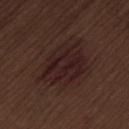The lesion was photographed on a routine skin check and not biopsied; there is no pathology result. The lesion-visualizer software estimated a footprint of about 20 mm², a shape eccentricity near 0.65, and two-axis asymmetry of about 0.25. It also reported a lesion-to-skin contrast of about 8 (normalized; higher = more distinct). And it measured a border-irregularity rating of about 3/10, a within-lesion color-variation index near 3.5/10, and radial color variation of about 1.5. Imaged with white-light lighting. The subject is a male about 70 years old. The lesion is located on the lower back. Longest diameter approximately 6.5 mm. A close-up tile cropped from a whole-body skin photograph, about 15 mm across.